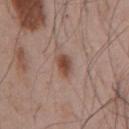Clinical impression:
This lesion was catalogued during total-body skin photography and was not selected for biopsy.
Context:
Imaged with white-light lighting. Longest diameter approximately 2.5 mm. Located on the chest. Cropped from a whole-body photographic skin survey; the tile spans about 15 mm. An algorithmic analysis of the crop reported an eccentricity of roughly 0.65. The software also gave a classifier nevus-likeness of about 95/100 and a detector confidence of about 100 out of 100 that the crop contains a lesion. A male subject in their mid-50s.